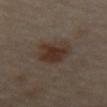| key | value |
|---|---|
| biopsy status | total-body-photography surveillance lesion; no biopsy |
| patient | female, aged 48 to 52 |
| size | about 4 mm |
| illumination | cross-polarized |
| anatomic site | the abdomen |
| automated lesion analysis | an area of roughly 12 mm², a shape eccentricity near 0.5, and a shape-asymmetry score of about 0.25 (0 = symmetric) |
| acquisition | ~15 mm crop, total-body skin-cancer survey |